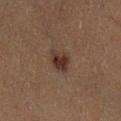Assessment: The lesion was tiled from a total-body skin photograph and was not biopsied. Acquisition and patient details: A female subject, in their 40s. This image is a 15 mm lesion crop taken from a total-body photograph. Automated tile analysis of the lesion measured a footprint of about 5 mm², an outline eccentricity of about 0.55 (0 = round, 1 = elongated), and a shape-asymmetry score of about 0.2 (0 = symmetric). The software also gave a lesion color around L≈26 a*≈15 b*≈20 in CIELAB, roughly 9 lightness units darker than nearby skin, and a lesion-to-skin contrast of about 9.5 (normalized; higher = more distinct). The analysis additionally found border irregularity of about 2 on a 0–10 scale, a within-lesion color-variation index near 3/10, and peripheral color asymmetry of about 1. The analysis additionally found an automated nevus-likeness rating near 85 out of 100 and a detector confidence of about 100 out of 100 that the crop contains a lesion. The lesion is located on the left lower leg. Measured at roughly 2.5 mm in maximum diameter.